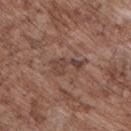No biopsy was performed on this lesion — it was imaged during a full skin examination and was not determined to be concerning. The lesion is located on the front of the torso. This is a white-light tile. About 4 mm across. Automated image analysis of the tile measured a border-irregularity rating of about 8.5/10 and peripheral color asymmetry of about 0.5. The software also gave lesion-presence confidence of about 75/100. This image is a 15 mm lesion crop taken from a total-body photograph. A male patient aged 68 to 72.